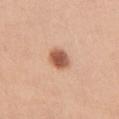patient: female, in their 30s | lesion diameter: ~3 mm (longest diameter) | TBP lesion metrics: an area of roughly 5.5 mm², a shape eccentricity near 0.65, and two-axis asymmetry of about 0.15; a border-irregularity rating of about 1.5/10 and internal color variation of about 3.5 on a 0–10 scale | site: the front of the torso | image: total-body-photography crop, ~15 mm field of view | tile lighting: white-light.